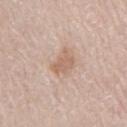Findings:
* notes: catalogued during a skin exam; not biopsied
* imaging modality: 15 mm crop, total-body photography
* lesion size: about 3 mm
* body site: the lower back
* lighting: white-light illumination
* subject: male, in their 80s